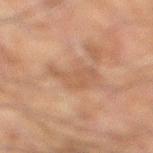Recorded during total-body skin imaging; not selected for excision or biopsy.
This image is a 15 mm lesion crop taken from a total-body photograph.
This is a cross-polarized tile.
The lesion's longest dimension is about 5.5 mm.
Located on the left leg.
A male subject approximately 60 years of age.